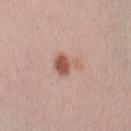Part of a total-body skin-imaging series; this lesion was reviewed on a skin check and was not flagged for biopsy. A male patient, aged approximately 35. The lesion is located on the left upper arm. The tile uses white-light illumination. Automated image analysis of the tile measured an outline eccentricity of about 0.6 (0 = round, 1 = elongated) and a symmetry-axis asymmetry near 0.4. The analysis additionally found a mean CIELAB color near L≈57 a*≈22 b*≈28, about 11 CIELAB-L* units darker than the surrounding skin, and a normalized border contrast of about 8. The analysis additionally found a border-irregularity index near 4/10, a color-variation rating of about 4/10, and radial color variation of about 1. And it measured an automated nevus-likeness rating near 90 out of 100 and a detector confidence of about 100 out of 100 that the crop contains a lesion. A region of skin cropped from a whole-body photographic capture, roughly 15 mm wide.